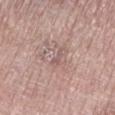Clinical impression: Recorded during total-body skin imaging; not selected for excision or biopsy. Clinical summary: Cropped from a total-body skin-imaging series; the visible field is about 15 mm. Imaged with white-light lighting. A female patient, approximately 70 years of age. Longest diameter approximately 3 mm. An algorithmic analysis of the crop reported a lesion area of about 2.5 mm², a shape eccentricity near 0.95, and a shape-asymmetry score of about 0.4 (0 = symmetric). From the leg.